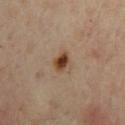| key | value |
|---|---|
| biopsy status | total-body-photography surveillance lesion; no biopsy |
| lesion diameter | ~2.5 mm (longest diameter) |
| anatomic site | the left upper arm |
| illumination | cross-polarized |
| acquisition | ~15 mm tile from a whole-body skin photo |
| TBP lesion metrics | a lesion area of about 4.5 mm², an eccentricity of roughly 0.7, and two-axis asymmetry of about 0.2; a border-irregularity index near 2/10 and peripheral color asymmetry of about 1.5 |
| subject | male, about 45 years old |